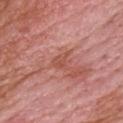Recorded during total-body skin imaging; not selected for excision or biopsy.
A male subject, aged 63 to 67.
Measured at roughly 2.5 mm in maximum diameter.
On the upper back.
A region of skin cropped from a whole-body photographic capture, roughly 15 mm wide.
The total-body-photography lesion software estimated a lesion area of about 4 mm² and a shape eccentricity near 0.65. It also reported a border-irregularity rating of about 5/10 and a within-lesion color-variation index near 2.5/10. And it measured a classifier nevus-likeness of about 0/100 and lesion-presence confidence of about 100/100.